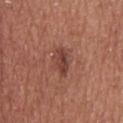workup — no biopsy performed (imaged during a skin exam); size — ≈4 mm; subject — male, aged 63–67; site — the upper back; image — 15 mm crop, total-body photography; illumination — white-light.